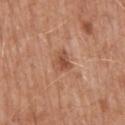Clinical impression:
This lesion was catalogued during total-body skin photography and was not selected for biopsy.
Acquisition and patient details:
Located on the mid back. The recorded lesion diameter is about 2.5 mm. A roughly 15 mm field-of-view crop from a total-body skin photograph. A male patient, aged 68–72. Automated image analysis of the tile measured a lesion color around L≈52 a*≈24 b*≈32 in CIELAB, roughly 9 lightness units darker than nearby skin, and a normalized lesion–skin contrast near 6.5. And it measured a classifier nevus-likeness of about 10/100 and a detector confidence of about 100 out of 100 that the crop contains a lesion.